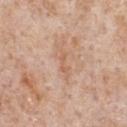No biopsy was performed on this lesion — it was imaged during a full skin examination and was not determined to be concerning. The lesion's longest dimension is about 2.5 mm. From the front of the torso. A 15 mm close-up tile from a total-body photography series done for melanoma screening. The total-body-photography lesion software estimated a border-irregularity rating of about 5/10, a within-lesion color-variation index near 0/10, and peripheral color asymmetry of about 0. And it measured a classifier nevus-likeness of about 0/100 and a lesion-detection confidence of about 100/100. The patient is a male in their mid- to late 60s.